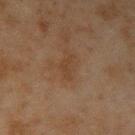Located on the right upper arm.
Imaged with cross-polarized lighting.
Automated tile analysis of the lesion measured an average lesion color of about L≈32 a*≈15 b*≈26 (CIELAB), roughly 4 lightness units darker than nearby skin, and a normalized lesion–skin contrast near 5. The software also gave a border-irregularity index near 6/10, a color-variation rating of about 0.5/10, and peripheral color asymmetry of about 0. It also reported an automated nevus-likeness rating near 0 out of 100 and lesion-presence confidence of about 100/100.
The lesion's longest dimension is about 3 mm.
A lesion tile, about 15 mm wide, cut from a 3D total-body photograph.
A male patient roughly 45 years of age.